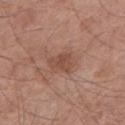Assessment:
Imaged during a routine full-body skin examination; the lesion was not biopsied and no histopathology is available.
Background:
A region of skin cropped from a whole-body photographic capture, roughly 15 mm wide. The lesion-visualizer software estimated a footprint of about 5 mm², a shape eccentricity near 0.65, and a shape-asymmetry score of about 0.35 (0 = symmetric). It also reported a lesion color around L≈48 a*≈21 b*≈27 in CIELAB and a normalized border contrast of about 6. And it measured a border-irregularity index near 3.5/10, internal color variation of about 2 on a 0–10 scale, and a peripheral color-asymmetry measure near 0.5. The software also gave a classifier nevus-likeness of about 0/100. Imaged with white-light lighting. Located on the left lower leg. A male patient, aged around 55. The lesion's longest dimension is about 2.5 mm.